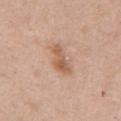notes=catalogued during a skin exam; not biopsied
image=15 mm crop, total-body photography
diameter=~4 mm (longest diameter)
subject=male, aged around 50
tile lighting=white-light
location=the arm